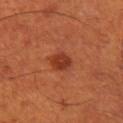Imaged during a routine full-body skin examination; the lesion was not biopsied and no histopathology is available. This is a cross-polarized tile. The subject is a male approximately 50 years of age. Measured at roughly 2.5 mm in maximum diameter. From the left thigh. A close-up tile cropped from a whole-body skin photograph, about 15 mm across.